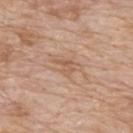Assessment:
Recorded during total-body skin imaging; not selected for excision or biopsy.
Background:
A 15 mm close-up tile from a total-body photography series done for melanoma screening. A male patient, aged approximately 80. Captured under white-light illumination. The lesion is on the upper back.